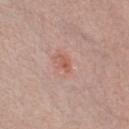A male patient aged approximately 50.
Imaged with white-light lighting.
The lesion is located on the chest.
The recorded lesion diameter is about 2 mm.
Cropped from a whole-body photographic skin survey; the tile spans about 15 mm.